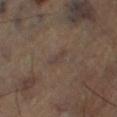Part of a total-body skin-imaging series; this lesion was reviewed on a skin check and was not flagged for biopsy.
The lesion's longest dimension is about 2.5 mm.
Cropped from a total-body skin-imaging series; the visible field is about 15 mm.
The tile uses cross-polarized illumination.
A male subject, about 65 years old.
On the left lower leg.